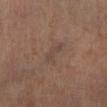Captured during whole-body skin photography for melanoma surveillance; the lesion was not biopsied.
The lesion's longest dimension is about 3 mm.
The subject is a female aged around 65.
Captured under cross-polarized illumination.
Cropped from a total-body skin-imaging series; the visible field is about 15 mm.
Automated image analysis of the tile measured a mean CIELAB color near L≈41 a*≈15 b*≈22 and about 5 CIELAB-L* units darker than the surrounding skin. It also reported border irregularity of about 4 on a 0–10 scale and peripheral color asymmetry of about 0. The analysis additionally found a classifier nevus-likeness of about 0/100 and lesion-presence confidence of about 100/100.
Located on the leg.